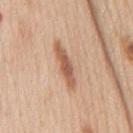Assessment:
Captured during whole-body skin photography for melanoma surveillance; the lesion was not biopsied.
Clinical summary:
The lesion-visualizer software estimated a lesion area of about 2.5 mm² and a symmetry-axis asymmetry near 0.25. The software also gave border irregularity of about 2.5 on a 0–10 scale, a within-lesion color-variation index near 0/10, and a peripheral color-asymmetry measure near 0. Cropped from a whole-body photographic skin survey; the tile spans about 15 mm. The patient is a male about 60 years old. On the mid back. The tile uses white-light illumination.